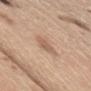<tbp_lesion>
  <biopsy_status>not biopsied; imaged during a skin examination</biopsy_status>
  <patient>
    <sex>male</sex>
    <age_approx>70</age_approx>
  </patient>
  <site>abdomen</site>
  <automated_metrics>
    <shape_asymmetry>0.2</shape_asymmetry>
    <vs_skin_darker_L>9.0</vs_skin_darker_L>
    <vs_skin_contrast_norm>6.0</vs_skin_contrast_norm>
    <border_irregularity_0_10>2.5</border_irregularity_0_10>
    <color_variation_0_10>1.5</color_variation_0_10>
  </automated_metrics>
  <lesion_size>
    <long_diameter_mm_approx>3.5</long_diameter_mm_approx>
  </lesion_size>
  <image>
    <source>total-body photography crop</source>
    <field_of_view_mm>15</field_of_view_mm>
  </image>
</tbp_lesion>